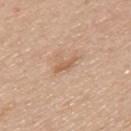Context:
A male subject, roughly 50 years of age. The lesion is on the mid back. The recorded lesion diameter is about 3 mm. A 15 mm close-up extracted from a 3D total-body photography capture. Imaged with white-light lighting. Automated tile analysis of the lesion measured about 9 CIELAB-L* units darker than the surrounding skin and a normalized border contrast of about 6.